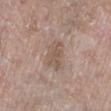{
  "biopsy_status": "not biopsied; imaged during a skin examination",
  "lighting": "white-light",
  "lesion_size": {
    "long_diameter_mm_approx": 4.0
  },
  "image": {
    "source": "total-body photography crop",
    "field_of_view_mm": 15
  },
  "patient": {
    "sex": "female",
    "age_approx": 65
  },
  "site": "leg"
}